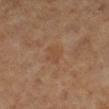Clinical impression:
The lesion was tiled from a total-body skin photograph and was not biopsied.